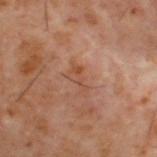The lesion was photographed on a routine skin check and not biopsied; there is no pathology result. Cropped from a total-body skin-imaging series; the visible field is about 15 mm. This is a cross-polarized tile. The lesion is located on the upper back. A male subject aged 58–62. The lesion-visualizer software estimated a nevus-likeness score of about 0/100 and lesion-presence confidence of about 100/100.